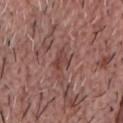biopsy status — catalogued during a skin exam; not biopsied
tile lighting — white-light
TBP lesion metrics — an area of roughly 3.5 mm², an outline eccentricity of about 0.6 (0 = round, 1 = elongated), and a symmetry-axis asymmetry near 0.35
size — ~2.5 mm (longest diameter)
patient — male, aged around 65
acquisition — ~15 mm tile from a whole-body skin photo
location — the chest